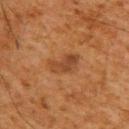notes: imaged on a skin check; not biopsied | anatomic site: the upper back | subject: male, in their 60s | imaging modality: ~15 mm tile from a whole-body skin photo.